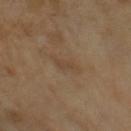| key | value |
|---|---|
| notes | no biopsy performed (imaged during a skin exam) |
| patient | female, roughly 55 years of age |
| acquisition | ~15 mm crop, total-body skin-cancer survey |
| anatomic site | the right upper arm |
| tile lighting | cross-polarized |
| automated metrics | an eccentricity of roughly 0.95 and a symmetry-axis asymmetry near 0.25; an average lesion color of about L≈44 a*≈14 b*≈30 (CIELAB), roughly 5 lightness units darker than nearby skin, and a normalized border contrast of about 5; a border-irregularity index near 3/10 and internal color variation of about 0 on a 0–10 scale; a nevus-likeness score of about 0/100 |
| lesion diameter | ≈3 mm |